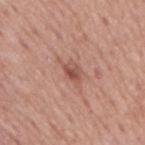  biopsy_status: not biopsied; imaged during a skin examination
  patient:
    sex: male
    age_approx: 75
  image:
    source: total-body photography crop
    field_of_view_mm: 15
  lighting: white-light
  site: mid back
  lesion_size:
    long_diameter_mm_approx: 3.0
  automated_metrics:
    eccentricity: 0.75
    shape_asymmetry: 0.25
    border_irregularity_0_10: 2.5
    peripheral_color_asymmetry: 2.0